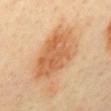<lesion>
  <biopsy_status>not biopsied; imaged during a skin examination</biopsy_status>
  <lighting>cross-polarized</lighting>
  <automated_metrics>
    <area_mm2_approx>25.0</area_mm2_approx>
    <eccentricity>0.75</eccentricity>
    <shape_asymmetry>0.2</shape_asymmetry>
    <nevus_likeness_0_100>85</nevus_likeness_0_100>
    <lesion_detection_confidence_0_100>100</lesion_detection_confidence_0_100>
  </automated_metrics>
  <lesion_size>
    <long_diameter_mm_approx>7.0</long_diameter_mm_approx>
  </lesion_size>
  <image>
    <source>total-body photography crop</source>
    <field_of_view_mm>15</field_of_view_mm>
  </image>
  <site>mid back</site>
  <patient>
    <sex>female</sex>
    <age_approx>45</age_approx>
  </patient>
</lesion>